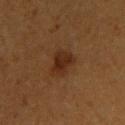<case>
  <biopsy_status>not biopsied; imaged during a skin examination</biopsy_status>
  <lesion_size>
    <long_diameter_mm_approx>3.5</long_diameter_mm_approx>
  </lesion_size>
  <patient>
    <sex>male</sex>
    <age_approx>55</age_approx>
  </patient>
  <site>chest</site>
  <lighting>cross-polarized</lighting>
  <image>
    <source>total-body photography crop</source>
    <field_of_view_mm>15</field_of_view_mm>
  </image>
</case>